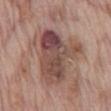workup = catalogued during a skin exam; not biopsied | patient = male, in their 70s | image-analysis metrics = a lesion–skin lightness drop of about 12 and a normalized lesion–skin contrast near 8.5; a border-irregularity rating of about 5/10 and peripheral color asymmetry of about 3; an automated nevus-likeness rating near 0 out of 100 and lesion-presence confidence of about 100/100 | tile lighting = white-light illumination | site = the abdomen | lesion diameter = about 7.5 mm | imaging modality = ~15 mm tile from a whole-body skin photo.